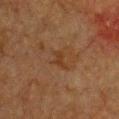Q: Was a biopsy performed?
A: no biopsy performed (imaged during a skin exam)
Q: How large is the lesion?
A: about 2.5 mm
Q: What is the anatomic site?
A: the chest
Q: Patient demographics?
A: female, roughly 55 years of age
Q: Illumination type?
A: cross-polarized
Q: What is the imaging modality?
A: total-body-photography crop, ~15 mm field of view